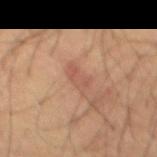Clinical impression: No biopsy was performed on this lesion — it was imaged during a full skin examination and was not determined to be concerning. Image and clinical context: The lesion is located on the mid back. The patient is a male roughly 65 years of age. Measured at roughly 3.5 mm in maximum diameter. Automated image analysis of the tile measured a mean CIELAB color near L≈49 a*≈21 b*≈27 and roughly 7 lightness units darker than nearby skin. The analysis additionally found a border-irregularity index near 4/10, a within-lesion color-variation index near 2/10, and peripheral color asymmetry of about 0.5. It also reported an automated nevus-likeness rating near 0 out of 100 and a lesion-detection confidence of about 100/100. This image is a 15 mm lesion crop taken from a total-body photograph. The tile uses cross-polarized illumination.